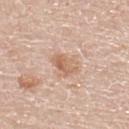follow-up = total-body-photography surveillance lesion; no biopsy | patient = male, aged 58–62 | location = the back | image = 15 mm crop, total-body photography.